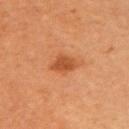This image is a 15 mm lesion crop taken from a total-body photograph. The total-body-photography lesion software estimated an area of roughly 5.5 mm² and a symmetry-axis asymmetry near 0.3. The software also gave a mean CIELAB color near L≈42 a*≈24 b*≈34, roughly 9 lightness units darker than nearby skin, and a normalized border contrast of about 7.5. The software also gave a border-irregularity rating of about 3/10 and radial color variation of about 1. The software also gave an automated nevus-likeness rating near 85 out of 100 and lesion-presence confidence of about 100/100. A female subject in their 60s. From the left upper arm.